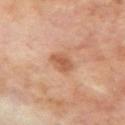Assessment:
The lesion was tiled from a total-body skin photograph and was not biopsied.
Image and clinical context:
The lesion is on the right upper arm. Measured at roughly 3 mm in maximum diameter. A 15 mm crop from a total-body photograph taken for skin-cancer surveillance. A male subject aged around 70. The lesion-visualizer software estimated a lesion area of about 5.5 mm² and an eccentricity of roughly 0.55. It also reported a classifier nevus-likeness of about 45/100 and lesion-presence confidence of about 100/100.